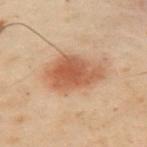<case>
<biopsy_status>not biopsied; imaged during a skin examination</biopsy_status>
<lesion_size>
  <long_diameter_mm_approx>5.5</long_diameter_mm_approx>
</lesion_size>
<site>arm</site>
<automated_metrics>
  <area_mm2_approx>17.0</area_mm2_approx>
  <eccentricity>0.65</eccentricity>
  <shape_asymmetry>0.2</shape_asymmetry>
  <cielab_L>45</cielab_L>
  <cielab_a>19</cielab_a>
  <cielab_b>29</cielab_b>
  <vs_skin_darker_L>10.0</vs_skin_darker_L>
  <vs_skin_contrast_norm>8.0</vs_skin_contrast_norm>
  <border_irregularity_0_10>2.0</border_irregularity_0_10>
  <color_variation_0_10>4.0</color_variation_0_10>
  <lesion_detection_confidence_0_100>100</lesion_detection_confidence_0_100>
</automated_metrics>
<patient>
  <sex>male</sex>
  <age_approx>55</age_approx>
</patient>
<image>
  <source>total-body photography crop</source>
  <field_of_view_mm>15</field_of_view_mm>
</image>
</case>